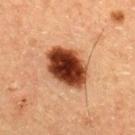No biopsy was performed on this lesion — it was imaged during a full skin examination and was not determined to be concerning. About 5.5 mm across. The tile uses cross-polarized illumination. An algorithmic analysis of the crop reported an area of roughly 17 mm² and two-axis asymmetry of about 0.15. It also reported a mean CIELAB color near L≈36 a*≈25 b*≈31, about 24 CIELAB-L* units darker than the surrounding skin, and a lesion-to-skin contrast of about 17.5 (normalized; higher = more distinct). It also reported a nevus-likeness score of about 95/100. A male patient, approximately 60 years of age. A close-up tile cropped from a whole-body skin photograph, about 15 mm across.